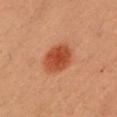Notes:
* follow-up: total-body-photography surveillance lesion; no biopsy
* image: total-body-photography crop, ~15 mm field of view
* patient: female, aged 38–42
* site: the chest